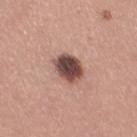Impression:
Captured during whole-body skin photography for melanoma surveillance; the lesion was not biopsied.
Clinical summary:
A female patient, aged 28 to 32. The total-body-photography lesion software estimated two-axis asymmetry of about 0.15. The analysis additionally found a lesion–skin lightness drop of about 20 and a normalized lesion–skin contrast near 13.5. The software also gave a border-irregularity rating of about 1.5/10, a within-lesion color-variation index near 7/10, and a peripheral color-asymmetry measure near 2. Cropped from a whole-body photographic skin survey; the tile spans about 15 mm. On the right thigh. Longest diameter approximately 3.5 mm. Captured under white-light illumination.